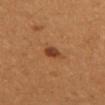Captured during whole-body skin photography for melanoma surveillance; the lesion was not biopsied. Imaged with cross-polarized lighting. A 15 mm crop from a total-body photograph taken for skin-cancer surveillance. A female subject, aged around 25. From the left upper arm. The lesion-visualizer software estimated a lesion-to-skin contrast of about 9 (normalized; higher = more distinct). It also reported an automated nevus-likeness rating near 100 out of 100 and a detector confidence of about 100 out of 100 that the crop contains a lesion.